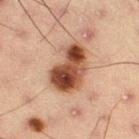body site = the left thigh
image source = ~15 mm crop, total-body skin-cancer survey
automated lesion analysis = border irregularity of about 3 on a 0–10 scale, a color-variation rating of about 9/10, and radial color variation of about 3.5; an automated nevus-likeness rating near 100 out of 100 and lesion-presence confidence of about 100/100
lesion diameter = ~6 mm (longest diameter)
illumination = cross-polarized
subject = male, roughly 55 years of age